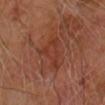biopsy status: total-body-photography surveillance lesion; no biopsy | patient: male, approximately 70 years of age | lesion size: about 4 mm | acquisition: ~15 mm crop, total-body skin-cancer survey | lighting: cross-polarized | body site: the right forearm.